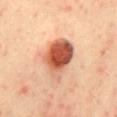A lesion tile, about 15 mm wide, cut from a 3D total-body photograph. The tile uses cross-polarized illumination. Located on the mid back. The subject is a female approximately 35 years of age.